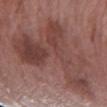Case summary:
* follow-up: imaged on a skin check; not biopsied
* automated metrics: a footprint of about 60 mm²; a classifier nevus-likeness of about 0/100 and a detector confidence of about 100 out of 100 that the crop contains a lesion
* subject: female, in their 60s
* lighting: white-light illumination
* location: the arm
* acquisition: total-body-photography crop, ~15 mm field of view